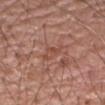{
  "site": "right upper arm",
  "automated_metrics": {
    "area_mm2_approx": 4.0,
    "eccentricity": 0.9,
    "shape_asymmetry": 0.35,
    "vs_skin_darker_L": 6.0,
    "vs_skin_contrast_norm": 5.5,
    "nevus_likeness_0_100": 0,
    "lesion_detection_confidence_0_100": 50
  },
  "image": {
    "source": "total-body photography crop",
    "field_of_view_mm": 15
  },
  "patient": {
    "sex": "male",
    "age_approx": 70
  }
}